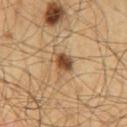Clinical impression:
Recorded during total-body skin imaging; not selected for excision or biopsy.
Image and clinical context:
A lesion tile, about 15 mm wide, cut from a 3D total-body photograph. Located on the mid back. The patient is a male aged 58 to 62.